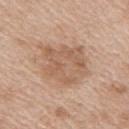  biopsy_status: not biopsied; imaged during a skin examination
  site: right upper arm
  lesion_size:
    long_diameter_mm_approx: 5.5
  automated_metrics:
    shape_asymmetry: 0.25
    border_irregularity_0_10: 3.0
    color_variation_0_10: 4.0
    peripheral_color_asymmetry: 1.5
    nevus_likeness_0_100: 10
    lesion_detection_confidence_0_100: 100
  image:
    source: total-body photography crop
    field_of_view_mm: 15
  patient:
    sex: female
    age_approx: 40
  lighting: white-light